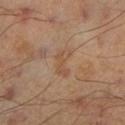A roughly 15 mm field-of-view crop from a total-body skin photograph. The patient is a male roughly 45 years of age. The tile uses cross-polarized illumination. From the left lower leg. The lesion's longest dimension is about 3.5 mm. The lesion-visualizer software estimated a footprint of about 4.5 mm², a shape eccentricity near 0.9, and a symmetry-axis asymmetry near 0.5. And it measured a border-irregularity index near 5.5/10 and a color-variation rating of about 1.5/10. And it measured a classifier nevus-likeness of about 0/100 and lesion-presence confidence of about 100/100.